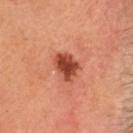patient = male, aged approximately 60
anatomic site = the head or neck
image source = total-body-photography crop, ~15 mm field of view
size = ≈4 mm
illumination = cross-polarized illumination
diagnosis = a compound melanocytic nevus (benign)